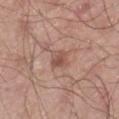No biopsy was performed on this lesion — it was imaged during a full skin examination and was not determined to be concerning. From the right lower leg. This image is a 15 mm lesion crop taken from a total-body photograph. Captured under white-light illumination. The patient is a male in their 40s.